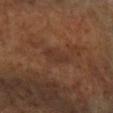Imaged during a routine full-body skin examination; the lesion was not biopsied and no histopathology is available. The recorded lesion diameter is about 3 mm. This is a cross-polarized tile. The lesion is located on the arm. A 15 mm crop from a total-body photograph taken for skin-cancer surveillance. A female subject, about 60 years old.